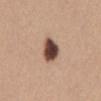biopsy status — total-body-photography surveillance lesion; no biopsy
imaging modality — ~15 mm tile from a whole-body skin photo
body site — the mid back
patient — female, aged 38 to 42